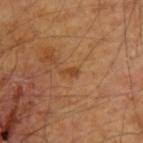Image and clinical context:
From the upper back. The lesion-visualizer software estimated an outline eccentricity of about 0.85 (0 = round, 1 = elongated). It also reported a mean CIELAB color near L≈42 a*≈22 b*≈37, about 8 CIELAB-L* units darker than the surrounding skin, and a normalized lesion–skin contrast near 7. The analysis additionally found a nevus-likeness score of about 0/100 and lesion-presence confidence of about 100/100. Captured under cross-polarized illumination. A male subject roughly 55 years of age. The lesion's longest dimension is about 2 mm. Cropped from a total-body skin-imaging series; the visible field is about 15 mm.